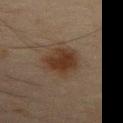Q: Was a biopsy performed?
A: no biopsy performed (imaged during a skin exam)
Q: What is the anatomic site?
A: the chest
Q: How was this image acquired?
A: ~15 mm tile from a whole-body skin photo
Q: Who is the patient?
A: male, in their mid-60s
Q: Automated lesion metrics?
A: an eccentricity of roughly 0.4 and a shape-asymmetry score of about 0.15 (0 = symmetric); an average lesion color of about L≈28 a*≈14 b*≈23 (CIELAB), about 8 CIELAB-L* units darker than the surrounding skin, and a lesion-to-skin contrast of about 9 (normalized; higher = more distinct); border irregularity of about 2 on a 0–10 scale, a color-variation rating of about 2.5/10, and a peripheral color-asymmetry measure near 1; a classifier nevus-likeness of about 95/100 and a detector confidence of about 100 out of 100 that the crop contains a lesion
Q: How large is the lesion?
A: about 4 mm